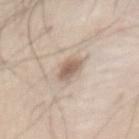Part of a total-body skin-imaging series; this lesion was reviewed on a skin check and was not flagged for biopsy.
From the abdomen.
A male patient roughly 45 years of age.
This image is a 15 mm lesion crop taken from a total-body photograph.
The tile uses white-light illumination.
An algorithmic analysis of the crop reported a lesion area of about 6.5 mm², a shape eccentricity near 0.75, and two-axis asymmetry of about 0.2. The analysis additionally found a border-irregularity rating of about 2.5/10, a within-lesion color-variation index near 4/10, and peripheral color asymmetry of about 1. It also reported a classifier nevus-likeness of about 75/100 and a lesion-detection confidence of about 95/100.
Approximately 3.5 mm at its widest.